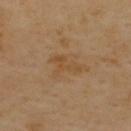<case>
  <biopsy_status>not biopsied; imaged during a skin examination</biopsy_status>
  <lighting>cross-polarized</lighting>
  <patient>
    <sex>male</sex>
    <age_approx>55</age_approx>
  </patient>
  <site>back</site>
  <image>
    <source>total-body photography crop</source>
    <field_of_view_mm>15</field_of_view_mm>
  </image>
  <lesion_size>
    <long_diameter_mm_approx>4.0</long_diameter_mm_approx>
  </lesion_size>
</case>